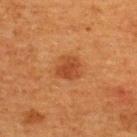{"biopsy_status": "not biopsied; imaged during a skin examination", "site": "upper back", "lesion_size": {"long_diameter_mm_approx": 3.0}, "patient": {"sex": "female", "age_approx": 40}, "image": {"source": "total-body photography crop", "field_of_view_mm": 15}, "lighting": "cross-polarized"}